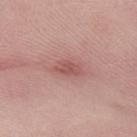follow-up: no biopsy performed (imaged during a skin exam)
image: total-body-photography crop, ~15 mm field of view
automated metrics: a lesion area of about 3.5 mm², a shape eccentricity near 0.75, and two-axis asymmetry of about 0.35; an average lesion color of about L≈53 a*≈26 b*≈23 (CIELAB); a classifier nevus-likeness of about 20/100 and lesion-presence confidence of about 100/100
lighting: white-light
anatomic site: the arm
subject: male, approximately 25 years of age